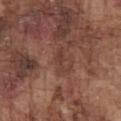Assessment: Imaged during a routine full-body skin examination; the lesion was not biopsied and no histopathology is available. Background: On the chest. Automated image analysis of the tile measured a footprint of about 3 mm² and a shape-asymmetry score of about 0.4 (0 = symmetric). It also reported an average lesion color of about L≈39 a*≈21 b*≈25 (CIELAB), roughly 6 lightness units darker than nearby skin, and a normalized border contrast of about 5.5. A male subject, in their mid- to late 70s. A lesion tile, about 15 mm wide, cut from a 3D total-body photograph.